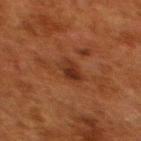workup: no biopsy performed (imaged during a skin exam) | size: ~3 mm (longest diameter) | lighting: cross-polarized illumination | patient: female, about 50 years old | location: the upper back | image source: total-body-photography crop, ~15 mm field of view | TBP lesion metrics: an outline eccentricity of about 0.55 (0 = round, 1 = elongated); a classifier nevus-likeness of about 40/100 and lesion-presence confidence of about 100/100.